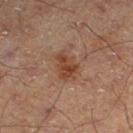Assessment:
No biopsy was performed on this lesion — it was imaged during a full skin examination and was not determined to be concerning.
Context:
A male patient, in their mid- to late 60s. Cropped from a total-body skin-imaging series; the visible field is about 15 mm. Longest diameter approximately 3.5 mm. The lesion is on the leg. The tile uses cross-polarized illumination.